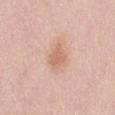Background: On the abdomen. The total-body-photography lesion software estimated a footprint of about 5 mm², an outline eccentricity of about 0.55 (0 = round, 1 = elongated), and a shape-asymmetry score of about 0.3 (0 = symmetric). The analysis additionally found a lesion-to-skin contrast of about 5.5 (normalized; higher = more distinct). And it measured a border-irregularity rating of about 2.5/10 and a within-lesion color-variation index near 2/10. It also reported a classifier nevus-likeness of about 60/100 and lesion-presence confidence of about 100/100. A 15 mm crop from a total-body photograph taken for skin-cancer surveillance. A female subject about 65 years old.